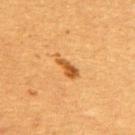Impression:
Captured during whole-body skin photography for melanoma surveillance; the lesion was not biopsied.